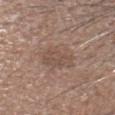- biopsy status — imaged on a skin check; not biopsied
- location — the head or neck
- image-analysis metrics — a border-irregularity rating of about 3/10, a within-lesion color-variation index near 3/10, and peripheral color asymmetry of about 1
- size — about 4 mm
- tile lighting — white-light
- subject — male, aged 48 to 52
- acquisition — total-body-photography crop, ~15 mm field of view